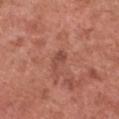This lesion was catalogued during total-body skin photography and was not selected for biopsy. Measured at roughly 2.5 mm in maximum diameter. A 15 mm crop from a total-body photograph taken for skin-cancer surveillance. The lesion is on the front of the torso. A female subject, aged around 50.